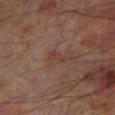follow-up: no biopsy performed (imaged during a skin exam)
acquisition: 15 mm crop, total-body photography
lighting: cross-polarized
anatomic site: the left lower leg
lesion size: about 2.5 mm
automated lesion analysis: a mean CIELAB color near L≈38 a*≈21 b*≈23 and about 5 CIELAB-L* units darker than the surrounding skin; an automated nevus-likeness rating near 20 out of 100
patient: male, about 70 years old